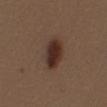Impression: The lesion was tiled from a total-body skin photograph and was not biopsied. Clinical summary: The lesion is located on the chest. A female patient about 40 years old. A region of skin cropped from a whole-body photographic capture, roughly 15 mm wide.